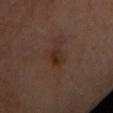{"biopsy_status": "not biopsied; imaged during a skin examination", "patient": {"sex": "female", "age_approx": 45}, "site": "upper back", "image": {"source": "total-body photography crop", "field_of_view_mm": 15}, "automated_metrics": {"area_mm2_approx": 4.0, "eccentricity": 0.7, "border_irregularity_0_10": 2.0, "color_variation_0_10": 3.5, "peripheral_color_asymmetry": 1.0}, "lesion_size": {"long_diameter_mm_approx": 2.5}, "lighting": "cross-polarized"}